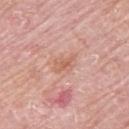Clinical summary:
Imaged with white-light lighting. A lesion tile, about 15 mm wide, cut from a 3D total-body photograph. The lesion is located on the upper back. A female patient, approximately 65 years of age.